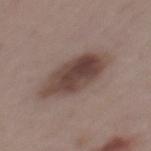<record>
<biopsy_status>not biopsied; imaged during a skin examination</biopsy_status>
<patient>
  <sex>female</sex>
  <age_approx>45</age_approx>
</patient>
<lighting>white-light</lighting>
<automated_metrics>
  <area_mm2_approx>21.0</area_mm2_approx>
  <eccentricity>0.85</eccentricity>
  <shape_asymmetry>0.15</shape_asymmetry>
  <nevus_likeness_0_100>70</nevus_likeness_0_100>
  <lesion_detection_confidence_0_100>100</lesion_detection_confidence_0_100>
</automated_metrics>
<site>mid back</site>
<image>
  <source>total-body photography crop</source>
  <field_of_view_mm>15</field_of_view_mm>
</image>
<lesion_size>
  <long_diameter_mm_approx>7.5</long_diameter_mm_approx>
</lesion_size>
</record>